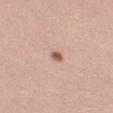Clinical impression:
The lesion was photographed on a routine skin check and not biopsied; there is no pathology result.
Background:
Longest diameter approximately 1.5 mm. A close-up tile cropped from a whole-body skin photograph, about 15 mm across. The lesion is on the leg. Automated tile analysis of the lesion measured a footprint of about 2 mm² and an outline eccentricity of about 0.6 (0 = round, 1 = elongated). It also reported a border-irregularity rating of about 2/10, a within-lesion color-variation index near 2/10, and radial color variation of about 0.5. It also reported an automated nevus-likeness rating near 95 out of 100. A female subject approximately 20 years of age.